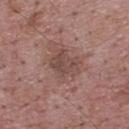• notes: total-body-photography surveillance lesion; no biopsy
• TBP lesion metrics: a footprint of about 8.5 mm², an eccentricity of roughly 0.6, and a symmetry-axis asymmetry near 0.3; an automated nevus-likeness rating near 0 out of 100 and lesion-presence confidence of about 100/100
• anatomic site: the upper back
• patient: male, about 70 years old
• acquisition: total-body-photography crop, ~15 mm field of view
• tile lighting: white-light
• lesion diameter: about 3.5 mm